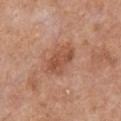Q: Is there a histopathology result?
A: no biopsy performed (imaged during a skin exam)
Q: How large is the lesion?
A: ~4.5 mm (longest diameter)
Q: Automated lesion metrics?
A: a lesion-to-skin contrast of about 7 (normalized; higher = more distinct); a lesion-detection confidence of about 100/100
Q: What is the anatomic site?
A: the chest
Q: How was the tile lit?
A: white-light illumination
Q: Patient demographics?
A: male, aged 58–62
Q: What is the imaging modality?
A: ~15 mm crop, total-body skin-cancer survey